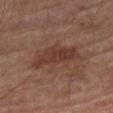Recorded during total-body skin imaging; not selected for excision or biopsy. A male patient in their 70s. On the leg. A close-up tile cropped from a whole-body skin photograph, about 15 mm across.